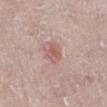biopsy_status: not biopsied; imaged during a skin examination
lesion_size:
  long_diameter_mm_approx: 2.5
site: leg
lighting: white-light
patient:
  sex: female
  age_approx: 70
image:
  source: total-body photography crop
  field_of_view_mm: 15
automated_metrics:
  area_mm2_approx: 4.5
  shape_asymmetry: 0.2
  vs_skin_darker_L: 8.0
  vs_skin_contrast_norm: 6.0
  nevus_likeness_0_100: 15
  lesion_detection_confidence_0_100: 100